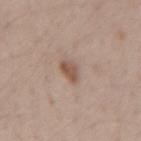Recorded during total-body skin imaging; not selected for excision or biopsy. On the left forearm. Approximately 2.5 mm at its widest. A close-up tile cropped from a whole-body skin photograph, about 15 mm across. The lesion-visualizer software estimated a lesion area of about 4 mm², an outline eccentricity of about 0.7 (0 = round, 1 = elongated), and two-axis asymmetry of about 0.25. The analysis additionally found a lesion color around L≈53 a*≈17 b*≈26 in CIELAB, about 11 CIELAB-L* units darker than the surrounding skin, and a lesion-to-skin contrast of about 7.5 (normalized; higher = more distinct). The analysis additionally found a border-irregularity index near 2/10, a within-lesion color-variation index near 2/10, and a peripheral color-asymmetry measure near 0.5. And it measured an automated nevus-likeness rating near 60 out of 100 and a lesion-detection confidence of about 100/100. A female patient, approximately 40 years of age. This is a white-light tile.